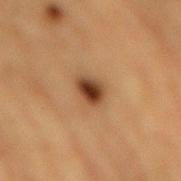follow-up = imaged on a skin check; not biopsied
tile lighting = cross-polarized
patient = male, aged 83 or over
TBP lesion metrics = border irregularity of about 2 on a 0–10 scale and peripheral color asymmetry of about 1.5; a lesion-detection confidence of about 100/100
diameter = ≈3 mm
location = the mid back
image = ~15 mm tile from a whole-body skin photo